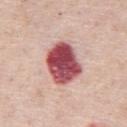Notes:
- notes · total-body-photography surveillance lesion; no biopsy
- diameter · about 5.5 mm
- imaging modality · 15 mm crop, total-body photography
- illumination · white-light
- anatomic site · the abdomen
- patient · male, approximately 75 years of age